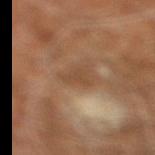Assessment: Imaged during a routine full-body skin examination; the lesion was not biopsied and no histopathology is available.